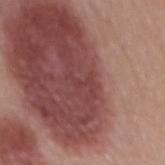Case summary:
- workup — no biopsy performed (imaged during a skin exam)
- image-analysis metrics — a lesion–skin lightness drop of about 5 and a lesion-to-skin contrast of about 4 (normalized; higher = more distinct); a border-irregularity rating of about 5.5/10, internal color variation of about 0 on a 0–10 scale, and a peripheral color-asymmetry measure near 0
- anatomic site — the mid back
- subject — male, aged 53–57
- tile lighting — white-light
- image — total-body-photography crop, ~15 mm field of view